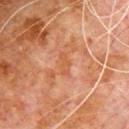This lesion was catalogued during total-body skin photography and was not selected for biopsy.
Located on the chest.
The lesion-visualizer software estimated a classifier nevus-likeness of about 0/100 and lesion-presence confidence of about 100/100.
The subject is a male aged approximately 80.
Measured at roughly 3 mm in maximum diameter.
A 15 mm crop from a total-body photograph taken for skin-cancer surveillance.